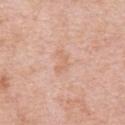Clinical impression:
Imaged during a routine full-body skin examination; the lesion was not biopsied and no histopathology is available.
Background:
A 15 mm close-up tile from a total-body photography series done for melanoma screening. From the arm. A male subject in their mid- to late 50s.